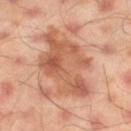biopsy_status: not biopsied; imaged during a skin examination
patient:
  sex: male
  age_approx: 45
image:
  source: total-body photography crop
  field_of_view_mm: 15
site: left thigh